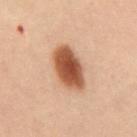Clinical summary: A male subject aged around 40. Captured under cross-polarized illumination. Located on the abdomen. A 15 mm close-up tile from a total-body photography series done for melanoma screening. Longest diameter approximately 5.5 mm.